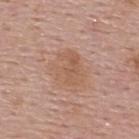Clinical impression: No biopsy was performed on this lesion — it was imaged during a full skin examination and was not determined to be concerning. Image and clinical context: From the upper back. A 15 mm crop from a total-body photograph taken for skin-cancer surveillance. Imaged with white-light lighting. The patient is a male in their mid- to late 50s. The lesion-visualizer software estimated about 6 CIELAB-L* units darker than the surrounding skin and a lesion-to-skin contrast of about 5.5 (normalized; higher = more distinct). It also reported a detector confidence of about 100 out of 100 that the crop contains a lesion. Longest diameter approximately 4 mm.